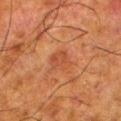follow-up: imaged on a skin check; not biopsied
TBP lesion metrics: a lesion area of about 5 mm² and a symmetry-axis asymmetry near 0.25; a lesion color around L≈39 a*≈24 b*≈31 in CIELAB and about 6 CIELAB-L* units darker than the surrounding skin; a nevus-likeness score of about 0/100 and lesion-presence confidence of about 100/100
tile lighting: cross-polarized illumination
acquisition: 15 mm crop, total-body photography
body site: the left lower leg
subject: male, aged 78 to 82